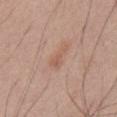biopsy status: no biopsy performed (imaged during a skin exam).